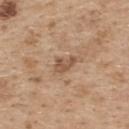| field | value |
|---|---|
| follow-up | catalogued during a skin exam; not biopsied |
| image | 15 mm crop, total-body photography |
| illumination | white-light illumination |
| body site | the upper back |
| subject | female, aged approximately 45 |
| diameter | ≈3 mm |
| TBP lesion metrics | a shape eccentricity near 0.8; a border-irregularity index near 3.5/10 |